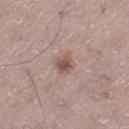No biopsy was performed on this lesion — it was imaged during a full skin examination and was not determined to be concerning. Located on the left thigh. A roughly 15 mm field-of-view crop from a total-body skin photograph. A male subject aged 63–67.